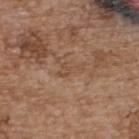Part of a total-body skin-imaging series; this lesion was reviewed on a skin check and was not flagged for biopsy. Measured at roughly 3 mm in maximum diameter. Captured under white-light illumination. From the upper back. A close-up tile cropped from a whole-body skin photograph, about 15 mm across. The subject is a female roughly 65 years of age.